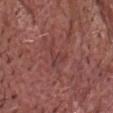Clinical impression: Recorded during total-body skin imaging; not selected for excision or biopsy. Clinical summary: The lesion is located on the chest. About 4 mm across. The subject is a male aged 73–77. Cropped from a whole-body photographic skin survey; the tile spans about 15 mm. The total-body-photography lesion software estimated a mean CIELAB color near L≈40 a*≈25 b*≈22, roughly 5 lightness units darker than nearby skin, and a normalized lesion–skin contrast near 4.5. The analysis additionally found lesion-presence confidence of about 55/100.